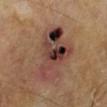| field | value |
|---|---|
| biopsy status | catalogued during a skin exam; not biopsied |
| lesion diameter | ~7.5 mm (longest diameter) |
| site | the left lower leg |
| subject | male, aged 63–67 |
| illumination | cross-polarized |
| automated metrics | a footprint of about 25 mm², an outline eccentricity of about 0.75 (0 = round, 1 = elongated), and a shape-asymmetry score of about 0.3 (0 = symmetric); border irregularity of about 4 on a 0–10 scale, a within-lesion color-variation index near 10/10, and peripheral color asymmetry of about 7; a classifier nevus-likeness of about 0/100 |
| imaging modality | 15 mm crop, total-body photography |